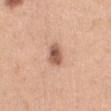Captured during whole-body skin photography for melanoma surveillance; the lesion was not biopsied.
A female subject about 30 years old.
The lesion is located on the chest.
The total-body-photography lesion software estimated a lesion-to-skin contrast of about 9 (normalized; higher = more distinct).
A roughly 15 mm field-of-view crop from a total-body skin photograph.
The recorded lesion diameter is about 3 mm.